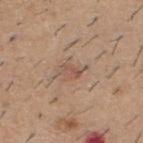subject = male, about 60 years old; tile lighting = white-light; lesion diameter = about 3 mm; anatomic site = the upper back; acquisition = ~15 mm crop, total-body skin-cancer survey.